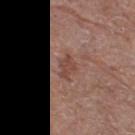Clinical impression: Recorded during total-body skin imaging; not selected for excision or biopsy. Clinical summary: A female patient aged approximately 80. The lesion is located on the right thigh. This is a white-light tile. Cropped from a whole-body photographic skin survey; the tile spans about 15 mm. The lesion's longest dimension is about 3 mm.